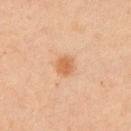Q: Is there a histopathology result?
A: imaged on a skin check; not biopsied
Q: Who is the patient?
A: female, aged approximately 45
Q: What kind of image is this?
A: 15 mm crop, total-body photography
Q: Illumination type?
A: cross-polarized
Q: Where on the body is the lesion?
A: the arm